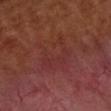Approximately 5 mm at its widest. Located on the left upper arm. Automated tile analysis of the lesion measured a mean CIELAB color near L≈34 a*≈27 b*≈24 and about 3 CIELAB-L* units darker than the surrounding skin. Captured under cross-polarized illumination. A female patient roughly 55 years of age. A lesion tile, about 15 mm wide, cut from a 3D total-body photograph.